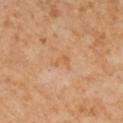biopsy status: imaged on a skin check; not biopsied
image-analysis metrics: an area of roughly 3 mm²; a border-irregularity rating of about 5/10, internal color variation of about 0.5 on a 0–10 scale, and peripheral color asymmetry of about 0; a classifier nevus-likeness of about 0/100 and a detector confidence of about 100 out of 100 that the crop contains a lesion
acquisition: ~15 mm crop, total-body skin-cancer survey
patient: female, about 50 years old
body site: the right lower leg
lesion size: ~2.5 mm (longest diameter)